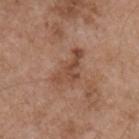Q: Is there a histopathology result?
A: no biopsy performed (imaged during a skin exam)
Q: What is the lesion's diameter?
A: ≈5 mm
Q: What is the imaging modality?
A: 15 mm crop, total-body photography
Q: How was the tile lit?
A: white-light
Q: Who is the patient?
A: male, aged 53 to 57
Q: Lesion location?
A: the front of the torso